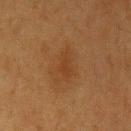Recorded during total-body skin imaging; not selected for excision or biopsy. An algorithmic analysis of the crop reported a lesion color around L≈32 a*≈18 b*≈30 in CIELAB, a lesion–skin lightness drop of about 5, and a normalized border contrast of about 5. Longest diameter approximately 3.5 mm. A 15 mm close-up tile from a total-body photography series done for melanoma screening. The lesion is located on the left upper arm. A female patient, roughly 40 years of age. The tile uses cross-polarized illumination.